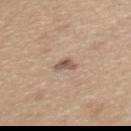Part of a total-body skin-imaging series; this lesion was reviewed on a skin check and was not flagged for biopsy.
A female subject approximately 45 years of age.
A 15 mm close-up tile from a total-body photography series done for melanoma screening.
The lesion is located on the upper back.